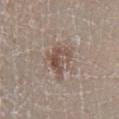Impression:
The lesion was photographed on a routine skin check and not biopsied; there is no pathology result.
Acquisition and patient details:
This is a white-light tile. On the right lower leg. A male subject, aged 58–62. The lesion's longest dimension is about 4 mm. Cropped from a total-body skin-imaging series; the visible field is about 15 mm.